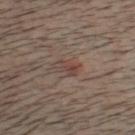{"biopsy_status": "not biopsied; imaged during a skin examination", "lesion_size": {"long_diameter_mm_approx": 3.0}, "image": {"source": "total-body photography crop", "field_of_view_mm": 15}, "patient": {"sex": "male", "age_approx": 55}, "site": "head or neck"}